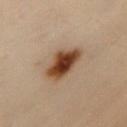| feature | finding |
|---|---|
| biopsy status | imaged on a skin check; not biopsied |
| patient | female, aged around 60 |
| image | ~15 mm tile from a whole-body skin photo |
| location | the chest |